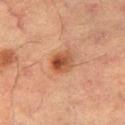  biopsy_status: not biopsied; imaged during a skin examination
  image:
    source: total-body photography crop
    field_of_view_mm: 15
  patient:
    sex: male
    age_approx: 65
  lighting: cross-polarized
  site: left thigh